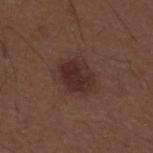A male patient approximately 50 years of age.
The lesion is on the back.
A 15 mm close-up tile from a total-body photography series done for melanoma screening.